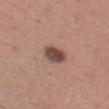The lesion was tiled from a total-body skin photograph and was not biopsied.
A female subject aged 28–32.
Longest diameter approximately 3 mm.
A 15 mm close-up tile from a total-body photography series done for melanoma screening.
Automated image analysis of the tile measured a footprint of about 6 mm², an outline eccentricity of about 0.7 (0 = round, 1 = elongated), and two-axis asymmetry of about 0.2. The analysis additionally found a nevus-likeness score of about 90/100 and lesion-presence confidence of about 100/100.
This is a white-light tile.
Located on the left thigh.